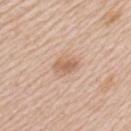Recorded during total-body skin imaging; not selected for excision or biopsy. On the left upper arm. Cropped from a total-body skin-imaging series; the visible field is about 15 mm. The patient is a female aged 43–47. This is a white-light tile. Longest diameter approximately 2.5 mm.